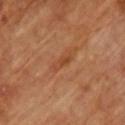This lesion was catalogued during total-body skin photography and was not selected for biopsy.
On the chest.
Automated image analysis of the tile measured peripheral color asymmetry of about 0.
A 15 mm close-up tile from a total-body photography series done for melanoma screening.
A male patient, about 65 years old.
This is a cross-polarized tile.
The recorded lesion diameter is about 3 mm.